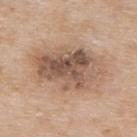Assessment: Captured during whole-body skin photography for melanoma surveillance; the lesion was not biopsied. Context: About 8.5 mm across. The lesion-visualizer software estimated a footprint of about 32 mm², an eccentricity of roughly 0.8, and a symmetry-axis asymmetry near 0.2. The software also gave a within-lesion color-variation index near 8.5/10 and a peripheral color-asymmetry measure near 3. It also reported a detector confidence of about 100 out of 100 that the crop contains a lesion. A male subject, about 65 years old. The lesion is on the upper back. Captured under white-light illumination. A close-up tile cropped from a whole-body skin photograph, about 15 mm across.